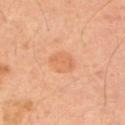<lesion>
  <biopsy_status>not biopsied; imaged during a skin examination</biopsy_status>
  <patient>
    <sex>male</sex>
    <age_approx>40</age_approx>
  </patient>
  <lesion_size>
    <long_diameter_mm_approx>3.0</long_diameter_mm_approx>
  </lesion_size>
  <lighting>cross-polarized</lighting>
  <site>left upper arm</site>
  <automated_metrics>
    <area_mm2_approx>7.0</area_mm2_approx>
    <eccentricity>0.4</eccentricity>
    <shape_asymmetry>0.15</shape_asymmetry>
    <nevus_likeness_0_100>35</nevus_likeness_0_100>
    <lesion_detection_confidence_0_100>100</lesion_detection_confidence_0_100>
  </automated_metrics>
  <image>
    <source>total-body photography crop</source>
    <field_of_view_mm>15</field_of_view_mm>
  </image>
</lesion>